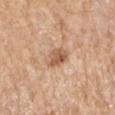Clinical impression:
The lesion was photographed on a routine skin check and not biopsied; there is no pathology result.
Background:
The patient is a female about 75 years old. From the right upper arm. Captured under white-light illumination. Cropped from a total-body skin-imaging series; the visible field is about 15 mm. The recorded lesion diameter is about 3 mm.